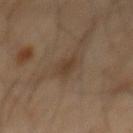Case summary:
- follow-up: catalogued during a skin exam; not biopsied
- image source: total-body-photography crop, ~15 mm field of view
- anatomic site: the back
- tile lighting: cross-polarized
- subject: male, in their 70s
- lesion size: about 3 mm